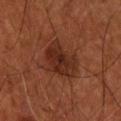biopsy status = catalogued during a skin exam; not biopsied